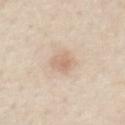The lesion was photographed on a routine skin check and not biopsied; there is no pathology result.
On the abdomen.
Longest diameter approximately 3.5 mm.
A male subject, aged approximately 60.
A 15 mm crop from a total-body photograph taken for skin-cancer surveillance.